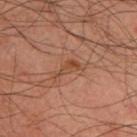Findings:
- follow-up · catalogued during a skin exam; not biopsied
- image-analysis metrics · an area of roughly 3.5 mm²; a lesion color around L≈46 a*≈23 b*≈32 in CIELAB, a lesion–skin lightness drop of about 8, and a normalized border contrast of about 6; a color-variation rating of about 2.5/10 and a peripheral color-asymmetry measure near 0.5; a nevus-likeness score of about 5/100 and a detector confidence of about 100 out of 100 that the crop contains a lesion
- size · ~3 mm (longest diameter)
- lighting · cross-polarized illumination
- image · 15 mm crop, total-body photography
- patient · male, aged 48–52
- location · the back